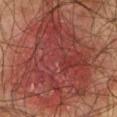This lesion was catalogued during total-body skin photography and was not selected for biopsy.